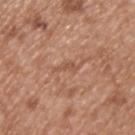{
  "biopsy_status": "not biopsied; imaged during a skin examination",
  "image": {
    "source": "total-body photography crop",
    "field_of_view_mm": 15
  },
  "site": "upper back",
  "automated_metrics": {
    "nevus_likeness_0_100": 0
  },
  "lighting": "white-light",
  "patient": {
    "sex": "male",
    "age_approx": 65
  }
}